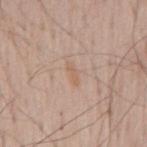notes — total-body-photography surveillance lesion; no biopsy
location — the mid back
lighting — white-light
patient — male, in their mid- to late 60s
diameter — ~3 mm (longest diameter)
imaging modality — ~15 mm tile from a whole-body skin photo